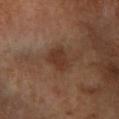{"site": "right lower leg", "image": {"source": "total-body photography crop", "field_of_view_mm": 15}, "patient": {"sex": "female", "age_approx": 65}}